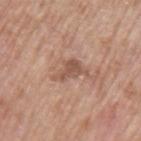Assessment: Captured during whole-body skin photography for melanoma surveillance; the lesion was not biopsied. Image and clinical context: The total-body-photography lesion software estimated an area of roughly 5.5 mm², an eccentricity of roughly 0.8, and a symmetry-axis asymmetry near 0.55. The software also gave a border-irregularity rating of about 6/10, a within-lesion color-variation index near 1.5/10, and radial color variation of about 0.5. Approximately 4 mm at its widest. This is a white-light tile. Located on the left upper arm. A male subject, aged 68–72. A roughly 15 mm field-of-view crop from a total-body skin photograph.